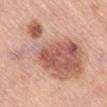Impression: Part of a total-body skin-imaging series; this lesion was reviewed on a skin check and was not flagged for biopsy. Context: The total-body-photography lesion software estimated a lesion color around L≈60 a*≈23 b*≈28 in CIELAB, roughly 12 lightness units darker than nearby skin, and a normalized border contrast of about 7.5. And it measured a classifier nevus-likeness of about 50/100 and lesion-presence confidence of about 100/100. The lesion is located on the mid back. The tile uses white-light illumination. A 15 mm close-up tile from a total-body photography series done for melanoma screening. Approximately 12.5 mm at its widest. A female patient in their mid- to late 60s.